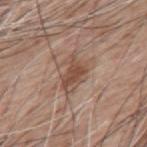This lesion was catalogued during total-body skin photography and was not selected for biopsy. Measured at roughly 4.5 mm in maximum diameter. On the mid back. A close-up tile cropped from a whole-body skin photograph, about 15 mm across. Imaged with white-light lighting. A male patient roughly 60 years of age.